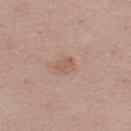Q: Was this lesion biopsied?
A: no biopsy performed (imaged during a skin exam)
Q: Lesion location?
A: the left thigh
Q: What are the patient's age and sex?
A: female, about 45 years old
Q: What is the imaging modality?
A: ~15 mm crop, total-body skin-cancer survey
Q: Automated lesion metrics?
A: an area of roughly 4 mm², an outline eccentricity of about 0.7 (0 = round, 1 = elongated), and a symmetry-axis asymmetry near 0.25; about 7 CIELAB-L* units darker than the surrounding skin; a color-variation rating of about 1/10 and radial color variation of about 0.5